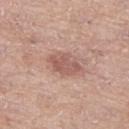follow-up — catalogued during a skin exam; not biopsied | location — the left thigh | acquisition — total-body-photography crop, ~15 mm field of view | subject — female, aged around 65.